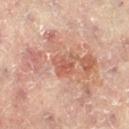{
  "biopsy_status": "not biopsied; imaged during a skin examination",
  "site": "left leg",
  "automated_metrics": {
    "eccentricity": 0.55,
    "cielab_L": 51,
    "cielab_a": 24,
    "cielab_b": 28,
    "vs_skin_contrast_norm": 5.5
  },
  "patient": {
    "sex": "female",
    "age_approx": 80
  },
  "lighting": "cross-polarized",
  "lesion_size": {
    "long_diameter_mm_approx": 3.0
  },
  "image": {
    "source": "total-body photography crop",
    "field_of_view_mm": 15
  }
}